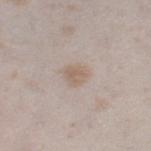Part of a total-body skin-imaging series; this lesion was reviewed on a skin check and was not flagged for biopsy. A region of skin cropped from a whole-body photographic capture, roughly 15 mm wide. Imaged with white-light lighting. The lesion is on the right thigh. The subject is a female aged approximately 25. Measured at roughly 2.5 mm in maximum diameter.